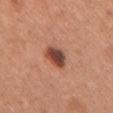No biopsy was performed on this lesion — it was imaged during a full skin examination and was not determined to be concerning.
A female subject, in their mid-50s.
Located on the chest.
The lesion's longest dimension is about 3.5 mm.
Imaged with white-light lighting.
A lesion tile, about 15 mm wide, cut from a 3D total-body photograph.